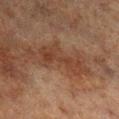Case summary:
• biopsy status · no biopsy performed (imaged during a skin exam)
• image · ~15 mm tile from a whole-body skin photo
• image-analysis metrics · a shape eccentricity near 0.9 and a shape-asymmetry score of about 0.55 (0 = symmetric); a lesion color around L≈32 a*≈18 b*≈25 in CIELAB, roughly 7 lightness units darker than nearby skin, and a normalized border contrast of about 6.5; a border-irregularity index near 8.5/10 and a color-variation rating of about 3/10; a nevus-likeness score of about 0/100 and a detector confidence of about 100 out of 100 that the crop contains a lesion
• location · the right lower leg
• lesion diameter · ~6 mm (longest diameter)
• tile lighting · cross-polarized illumination
• patient · male, aged around 70